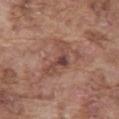site: abdomen
patient:
  sex: male
  age_approx: 75
lighting: white-light
image:
  source: total-body photography crop
  field_of_view_mm: 15
lesion_size:
  long_diameter_mm_approx: 5.0
automated_metrics:
  area_mm2_approx: 8.5
  eccentricity: 0.85
  shape_asymmetry: 0.6
  cielab_L: 46
  cielab_a: 21
  cielab_b: 25
  vs_skin_contrast_norm: 7.0
  border_irregularity_0_10: 6.5
  peripheral_color_asymmetry: 1.5